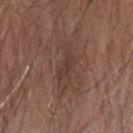The lesion was tiled from a total-body skin photograph and was not biopsied.
Cropped from a whole-body photographic skin survey; the tile spans about 15 mm.
A male subject aged 73 to 77.
The lesion is located on the arm.
Imaged with white-light lighting.